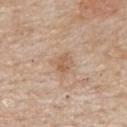<lesion>
  <biopsy_status>not biopsied; imaged during a skin examination</biopsy_status>
  <site>mid back</site>
  <image>
    <source>total-body photography crop</source>
    <field_of_view_mm>15</field_of_view_mm>
  </image>
  <automated_metrics>
    <vs_skin_darker_L>8.0</vs_skin_darker_L>
  </automated_metrics>
  <patient>
    <sex>male</sex>
    <age_approx>55</age_approx>
  </patient>
</lesion>